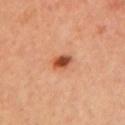notes: imaged on a skin check; not biopsied
image source: ~15 mm crop, total-body skin-cancer survey
subject: female, aged 33–37
site: the arm